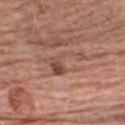| field | value |
|---|---|
| notes | catalogued during a skin exam; not biopsied |
| image source | ~15 mm crop, total-body skin-cancer survey |
| lesion size | about 5 mm |
| automated metrics | an area of roughly 8 mm², an eccentricity of roughly 0.9, and two-axis asymmetry of about 0.75; radial color variation of about 2; a classifier nevus-likeness of about 5/100 and a lesion-detection confidence of about 100/100 |
| illumination | white-light |
| subject | male, approximately 80 years of age |
| site | the chest |